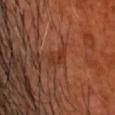Imaged during a routine full-body skin examination; the lesion was not biopsied and no histopathology is available. The tile uses cross-polarized illumination. About 3 mm across. The lesion is located on the head or neck. The patient is a male aged around 50. A close-up tile cropped from a whole-body skin photograph, about 15 mm across.